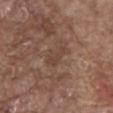Captured during whole-body skin photography for melanoma surveillance; the lesion was not biopsied. A region of skin cropped from a whole-body photographic capture, roughly 15 mm wide. Imaged with white-light lighting. The lesion is on the mid back. A male subject aged 78 to 82. Approximately 3.5 mm at its widest.